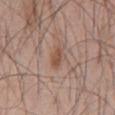Recorded during total-body skin imaging; not selected for excision or biopsy.